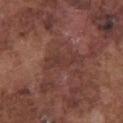Clinical summary: From the front of the torso. Captured under white-light illumination. A male patient in their mid-70s. The lesion's longest dimension is about 3.5 mm. A region of skin cropped from a whole-body photographic capture, roughly 15 mm wide.